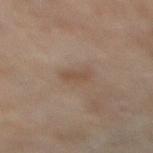The lesion was photographed on a routine skin check and not biopsied; there is no pathology result. The total-body-photography lesion software estimated a mean CIELAB color near L≈43 a*≈14 b*≈25, roughly 6 lightness units darker than nearby skin, and a normalized border contrast of about 5.5. About 3 mm across. From the right lower leg. The tile uses cross-polarized illumination. The subject is a female aged approximately 60. A 15 mm close-up extracted from a 3D total-body photography capture.